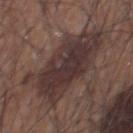Image and clinical context:
A close-up tile cropped from a whole-body skin photograph, about 15 mm across. Imaged with white-light lighting. The total-body-photography lesion software estimated a lesion color around L≈34 a*≈15 b*≈17 in CIELAB and a normalized lesion–skin contrast near 9.5. Approximately 9.5 mm at its widest. On the chest. The subject is a male aged 73 to 77.